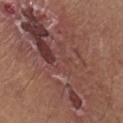Assessment: Imaged during a routine full-body skin examination; the lesion was not biopsied and no histopathology is available. Image and clinical context: The lesion is located on the right lower leg. A male subject in their mid- to late 60s. The tile uses white-light illumination. A 15 mm crop from a total-body photograph taken for skin-cancer surveillance. The lesion-visualizer software estimated a shape eccentricity near 0.85 and a shape-asymmetry score of about 0.35 (0 = symmetric). The software also gave an average lesion color of about L≈44 a*≈21 b*≈22 (CIELAB), about 8 CIELAB-L* units darker than the surrounding skin, and a lesion-to-skin contrast of about 7 (normalized; higher = more distinct). The analysis additionally found a detector confidence of about 75 out of 100 that the crop contains a lesion.